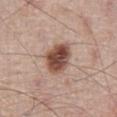This lesion was catalogued during total-body skin photography and was not selected for biopsy.
An algorithmic analysis of the crop reported an area of roughly 10 mm² and a symmetry-axis asymmetry near 0.15. And it measured border irregularity of about 1.5 on a 0–10 scale, internal color variation of about 5.5 on a 0–10 scale, and a peripheral color-asymmetry measure near 1.5. The analysis additionally found an automated nevus-likeness rating near 100 out of 100 and lesion-presence confidence of about 100/100.
This image is a 15 mm lesion crop taken from a total-body photograph.
The lesion is on the abdomen.
This is a white-light tile.
The patient is a male roughly 65 years of age.